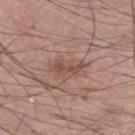Case summary:
• biopsy status · catalogued during a skin exam; not biopsied
• patient · male, aged 58–62
• lesion size · ≈3.5 mm
• imaging modality · 15 mm crop, total-body photography
• site · the right lower leg
• automated metrics · a shape eccentricity near 0.95 and a shape-asymmetry score of about 0.4 (0 = symmetric)
• illumination · white-light